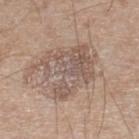follow-up — no biopsy performed (imaged during a skin exam); body site — the left lower leg; subject — male, aged 63 to 67; acquisition — ~15 mm tile from a whole-body skin photo.